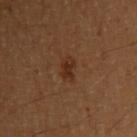Clinical impression:
Imaged during a routine full-body skin examination; the lesion was not biopsied and no histopathology is available.
Background:
The lesion is located on the right upper arm. A 15 mm close-up extracted from a 3D total-body photography capture. A female subject aged approximately 50. Imaged with cross-polarized lighting. Automated tile analysis of the lesion measured an eccentricity of roughly 0.7 and two-axis asymmetry of about 0.3. And it measured a mean CIELAB color near L≈25 a*≈17 b*≈25 and a lesion–skin lightness drop of about 6. The software also gave lesion-presence confidence of about 100/100. About 2.5 mm across.